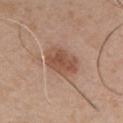Notes:
• subject — male, aged 48–52
• image source — total-body-photography crop, ~15 mm field of view
• site — the chest